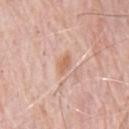Impression: Part of a total-body skin-imaging series; this lesion was reviewed on a skin check and was not flagged for biopsy. Acquisition and patient details: Located on the chest. A male subject aged around 80. Imaged with white-light lighting. A roughly 15 mm field-of-view crop from a total-body skin photograph. The total-body-photography lesion software estimated a lesion area of about 3.5 mm², an outline eccentricity of about 0.7 (0 = round, 1 = elongated), and a shape-asymmetry score of about 0.15 (0 = symmetric). The software also gave an average lesion color of about L≈64 a*≈21 b*≈32 (CIELAB), about 9 CIELAB-L* units darker than the surrounding skin, and a normalized lesion–skin contrast near 6.5.